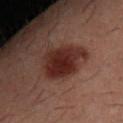• workup — total-body-photography surveillance lesion; no biopsy
• lesion diameter — about 5 mm
• anatomic site — the arm
• image-analysis metrics — a border-irregularity rating of about 2.5/10, a color-variation rating of about 4/10, and peripheral color asymmetry of about 1.5
• subject — male, aged 28–32
• imaging modality — ~15 mm crop, total-body skin-cancer survey
• lighting — cross-polarized